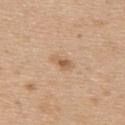notes=total-body-photography surveillance lesion; no biopsy | imaging modality=total-body-photography crop, ~15 mm field of view | lighting=white-light illumination | patient=female, aged around 45 | lesion diameter=~2.5 mm (longest diameter) | image-analysis metrics=a lesion color around L≈60 a*≈19 b*≈35 in CIELAB, a lesion–skin lightness drop of about 9, and a normalized lesion–skin contrast near 6.5; an automated nevus-likeness rating near 35 out of 100 | site=the upper back.